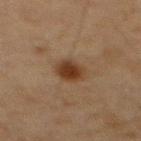Clinical impression:
The lesion was tiled from a total-body skin photograph and was not biopsied.
Clinical summary:
A close-up tile cropped from a whole-body skin photograph, about 15 mm across. The subject is a male aged approximately 75. Measured at roughly 3 mm in maximum diameter. The lesion is located on the abdomen. The tile uses cross-polarized illumination. The lesion-visualizer software estimated a mean CIELAB color near L≈30 a*≈16 b*≈26, roughly 10 lightness units darker than nearby skin, and a normalized border contrast of about 10. It also reported border irregularity of about 1 on a 0–10 scale and a peripheral color-asymmetry measure near 1. And it measured a classifier nevus-likeness of about 95/100 and lesion-presence confidence of about 100/100.